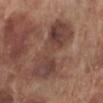The lesion was tiled from a total-body skin photograph and was not biopsied. A male subject, aged 68 to 72. The total-body-photography lesion software estimated a border-irregularity index near 5.5/10, a within-lesion color-variation index near 5.5/10, and radial color variation of about 1.5. The analysis additionally found an automated nevus-likeness rating near 0 out of 100 and a detector confidence of about 100 out of 100 that the crop contains a lesion. From the left lower leg. Imaged with white-light lighting. This image is a 15 mm lesion crop taken from a total-body photograph.